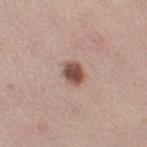Imaged during a routine full-body skin examination; the lesion was not biopsied and no histopathology is available.
A female subject roughly 45 years of age.
Located on the right thigh.
Captured under white-light illumination.
A lesion tile, about 15 mm wide, cut from a 3D total-body photograph.